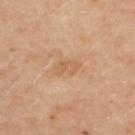Case summary:
- workup — total-body-photography surveillance lesion; no biopsy
- body site — the chest
- subject — female, roughly 50 years of age
- automated metrics — a lesion color around L≈57 a*≈20 b*≈35 in CIELAB and about 6 CIELAB-L* units darker than the surrounding skin; a border-irregularity index near 2.5/10, internal color variation of about 2.5 on a 0–10 scale, and a peripheral color-asymmetry measure near 1
- imaging modality — ~15 mm crop, total-body skin-cancer survey